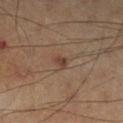Impression:
The lesion was tiled from a total-body skin photograph and was not biopsied.
Background:
The recorded lesion diameter is about 1.5 mm. A region of skin cropped from a whole-body photographic capture, roughly 15 mm wide. The total-body-photography lesion software estimated a lesion area of about 2 mm², an outline eccentricity of about 0.6 (0 = round, 1 = elongated), and a shape-asymmetry score of about 0.4 (0 = symmetric). The analysis additionally found a lesion color around L≈38 a*≈17 b*≈24 in CIELAB, a lesion–skin lightness drop of about 9, and a lesion-to-skin contrast of about 7.5 (normalized; higher = more distinct). The analysis additionally found an automated nevus-likeness rating near 50 out of 100 and a lesion-detection confidence of about 100/100. Imaged with cross-polarized lighting. On the left lower leg. A male subject aged 58 to 62.